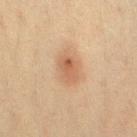Imaged during a routine full-body skin examination; the lesion was not biopsied and no histopathology is available.
A close-up tile cropped from a whole-body skin photograph, about 15 mm across.
Longest diameter approximately 3 mm.
Automated image analysis of the tile measured an area of roughly 6 mm² and an eccentricity of roughly 0.75. It also reported a border-irregularity index near 1.5/10, internal color variation of about 4.5 on a 0–10 scale, and radial color variation of about 1.5. It also reported lesion-presence confidence of about 100/100.
On the leg.
A female patient aged 38–42.